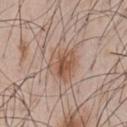Impression: Imaged during a routine full-body skin examination; the lesion was not biopsied and no histopathology is available. Clinical summary: The lesion is located on the chest. A 15 mm close-up extracted from a 3D total-body photography capture. The subject is a male aged around 50. The total-body-photography lesion software estimated a mean CIELAB color near L≈55 a*≈19 b*≈29 and a normalized lesion–skin contrast near 7.5. The analysis additionally found border irregularity of about 3 on a 0–10 scale, a color-variation rating of about 6.5/10, and peripheral color asymmetry of about 2.5.